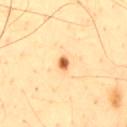notes: total-body-photography surveillance lesion; no biopsy
patient: male, roughly 45 years of age
image-analysis metrics: a footprint of about 2.5 mm², a shape eccentricity near 0.65, and a shape-asymmetry score of about 0.3 (0 = symmetric); a mean CIELAB color near L≈67 a*≈26 b*≈45, about 18 CIELAB-L* units darker than the surrounding skin, and a normalized lesion–skin contrast near 10.5; border irregularity of about 2.5 on a 0–10 scale and a peripheral color-asymmetry measure near 1.5
acquisition: total-body-photography crop, ~15 mm field of view
anatomic site: the mid back
tile lighting: cross-polarized illumination